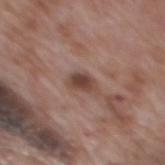Recorded during total-body skin imaging; not selected for excision or biopsy. Captured under white-light illumination. The lesion is located on the mid back. A lesion tile, about 15 mm wide, cut from a 3D total-body photograph. Approximately 3 mm at its widest. A male patient roughly 70 years of age. Automated tile analysis of the lesion measured an area of roughly 4 mm², an outline eccentricity of about 0.75 (0 = round, 1 = elongated), and a symmetry-axis asymmetry near 0.25. It also reported a normalized lesion–skin contrast near 9.5. The analysis additionally found border irregularity of about 2.5 on a 0–10 scale and peripheral color asymmetry of about 1.